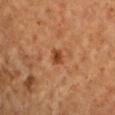{"biopsy_status": "not biopsied; imaged during a skin examination", "patient": {"sex": "female", "age_approx": 70}, "lighting": "cross-polarized", "image": {"source": "total-body photography crop", "field_of_view_mm": 15}, "automated_metrics": {"cielab_L": 43, "cielab_a": 26, "cielab_b": 37, "vs_skin_darker_L": 11.0, "vs_skin_contrast_norm": 8.5, "border_irregularity_0_10": 2.5, "color_variation_0_10": 2.0}, "site": "arm"}